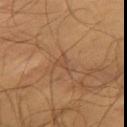The lesion was photographed on a routine skin check and not biopsied; there is no pathology result. Captured under cross-polarized illumination. From the chest. The lesion-visualizer software estimated a border-irregularity rating of about 6.5/10 and a color-variation rating of about 1/10. A region of skin cropped from a whole-body photographic capture, roughly 15 mm wide. The lesion's longest dimension is about 2.5 mm. A male patient, roughly 55 years of age.